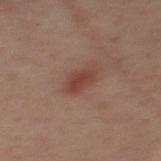The lesion was tiled from a total-body skin photograph and was not biopsied.
A roughly 15 mm field-of-view crop from a total-body skin photograph.
Captured under cross-polarized illumination.
On the back.
A male subject roughly 40 years of age.
About 4 mm across.